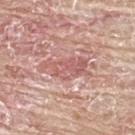biopsy status: total-body-photography surveillance lesion; no biopsy
location: the upper back
acquisition: ~15 mm tile from a whole-body skin photo
TBP lesion metrics: an area of roughly 9.5 mm² and two-axis asymmetry of about 0.4; a mean CIELAB color near L≈59 a*≈26 b*≈24, a lesion–skin lightness drop of about 9, and a normalized border contrast of about 6; a classifier nevus-likeness of about 0/100
illumination: white-light illumination
size: ~5.5 mm (longest diameter)
patient: male, aged approximately 65